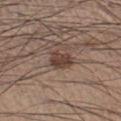Assessment:
The lesion was photographed on a routine skin check and not biopsied; there is no pathology result.
Clinical summary:
The lesion is on the left lower leg. A close-up tile cropped from a whole-body skin photograph, about 15 mm across. This is a white-light tile. Automated tile analysis of the lesion measured an area of roughly 5.5 mm², an outline eccentricity of about 0.75 (0 = round, 1 = elongated), and a shape-asymmetry score of about 0.25 (0 = symmetric). The software also gave a classifier nevus-likeness of about 85/100 and a detector confidence of about 100 out of 100 that the crop contains a lesion. Approximately 3 mm at its widest. A male subject approximately 35 years of age.